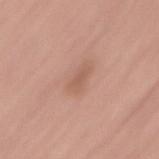No biopsy was performed on this lesion — it was imaged during a full skin examination and was not determined to be concerning. The patient is a male aged 63–67. From the lower back. The tile uses white-light illumination. A roughly 15 mm field-of-view crop from a total-body skin photograph.